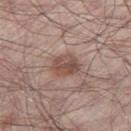notes = total-body-photography surveillance lesion; no biopsy